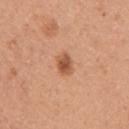Case summary:
• notes — total-body-photography surveillance lesion; no biopsy
• site — the right upper arm
• subject — female, aged 43 to 47
• diameter — ~2.5 mm (longest diameter)
• acquisition — total-body-photography crop, ~15 mm field of view
• TBP lesion metrics — an area of roughly 4 mm², an outline eccentricity of about 0.65 (0 = round, 1 = elongated), and a symmetry-axis asymmetry near 0.2; a lesion-to-skin contrast of about 8 (normalized; higher = more distinct); a border-irregularity rating of about 1.5/10 and radial color variation of about 1; a classifier nevus-likeness of about 95/100
• illumination — white-light